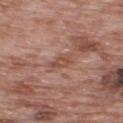No biopsy was performed on this lesion — it was imaged during a full skin examination and was not determined to be concerning.
On the upper back.
The recorded lesion diameter is about 3 mm.
This is a white-light tile.
A male subject, roughly 70 years of age.
A 15 mm crop from a total-body photograph taken for skin-cancer surveillance.
The lesion-visualizer software estimated an average lesion color of about L≈50 a*≈22 b*≈28 (CIELAB) and a normalized border contrast of about 6. The analysis additionally found a border-irregularity index near 3/10 and peripheral color asymmetry of about 1.